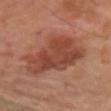Assessment:
Recorded during total-body skin imaging; not selected for excision or biopsy.
Acquisition and patient details:
A close-up tile cropped from a whole-body skin photograph, about 15 mm across. The lesion-visualizer software estimated an outline eccentricity of about 0.75 (0 = round, 1 = elongated) and two-axis asymmetry of about 0.35. The software also gave border irregularity of about 4 on a 0–10 scale and a color-variation rating of about 4/10. This is a cross-polarized tile. On the arm. A male patient, aged 68–72. About 7.5 mm across.